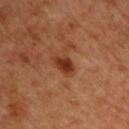• biopsy status: catalogued during a skin exam; not biopsied
• tile lighting: cross-polarized illumination
• imaging modality: ~15 mm tile from a whole-body skin photo
• body site: the front of the torso
• subject: male, aged approximately 50
• automated lesion analysis: an area of roughly 4.5 mm² and a shape-asymmetry score of about 0.25 (0 = symmetric); internal color variation of about 3 on a 0–10 scale and a peripheral color-asymmetry measure near 1; a nevus-likeness score of about 95/100 and lesion-presence confidence of about 100/100
• size: about 3 mm